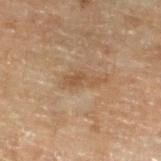Notes:
– notes — imaged on a skin check; not biopsied
– body site — the left lower leg
– illumination — cross-polarized illumination
– image — total-body-photography crop, ~15 mm field of view
– automated metrics — an area of roughly 4 mm² and an outline eccentricity of about 0.95 (0 = round, 1 = elongated); an average lesion color of about L≈39 a*≈13 b*≈27 (CIELAB), a lesion–skin lightness drop of about 5, and a lesion-to-skin contrast of about 5.5 (normalized; higher = more distinct); a nevus-likeness score of about 0/100 and a lesion-detection confidence of about 95/100
– subject — male, aged 68 to 72
– diameter — about 4 mm